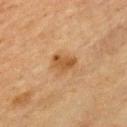Impression:
The lesion was photographed on a routine skin check and not biopsied; there is no pathology result.
Clinical summary:
Located on the chest. About 3 mm across. The patient is a male roughly 45 years of age. A roughly 15 mm field-of-view crop from a total-body skin photograph.